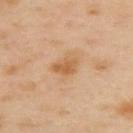The lesion was tiled from a total-body skin photograph and was not biopsied.
A roughly 15 mm field-of-view crop from a total-body skin photograph.
The lesion's longest dimension is about 3 mm.
The patient is a female aged 38–42.
This is a cross-polarized tile.
The total-body-photography lesion software estimated a footprint of about 5 mm² and a symmetry-axis asymmetry near 0.3. The software also gave a border-irregularity index near 3/10 and a color-variation rating of about 3/10. The analysis additionally found a classifier nevus-likeness of about 5/100 and lesion-presence confidence of about 100/100.
From the upper back.